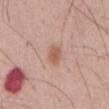| key | value |
|---|---|
| workup | catalogued during a skin exam; not biopsied |
| acquisition | total-body-photography crop, ~15 mm field of view |
| tile lighting | white-light illumination |
| automated metrics | a footprint of about 4 mm², an outline eccentricity of about 0.7 (0 = round, 1 = elongated), and a symmetry-axis asymmetry near 0.25; border irregularity of about 2 on a 0–10 scale and peripheral color asymmetry of about 0.5; a classifier nevus-likeness of about 90/100 and a lesion-detection confidence of about 100/100 |
| subject | male, aged approximately 55 |